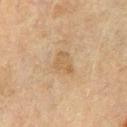The lesion was tiled from a total-body skin photograph and was not biopsied.
From the front of the torso.
A male subject aged around 70.
About 3 mm across.
The tile uses cross-polarized illumination.
A region of skin cropped from a whole-body photographic capture, roughly 15 mm wide.